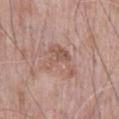| key | value |
|---|---|
| biopsy status | catalogued during a skin exam; not biopsied |
| site | the front of the torso |
| subject | male, aged 68–72 |
| image | 15 mm crop, total-body photography |